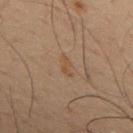The lesion was tiled from a total-body skin photograph and was not biopsied.